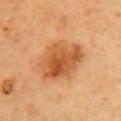| feature | finding |
|---|---|
| notes | no biopsy performed (imaged during a skin exam) |
| image | ~15 mm crop, total-body skin-cancer survey |
| size | about 6 mm |
| tile lighting | cross-polarized |
| location | the chest |
| subject | female, aged 43 to 47 |